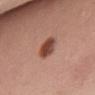Captured during whole-body skin photography for melanoma surveillance; the lesion was not biopsied. A female subject, aged around 50. An algorithmic analysis of the crop reported a lesion color around L≈46 a*≈25 b*≈29 in CIELAB, about 15 CIELAB-L* units darker than the surrounding skin, and a normalized lesion–skin contrast near 10.5. It also reported a border-irregularity index near 1.5/10 and a peripheral color-asymmetry measure near 1.5. A 15 mm crop from a total-body photograph taken for skin-cancer surveillance. Approximately 3.5 mm at its widest. The tile uses white-light illumination. The lesion is on the front of the torso.